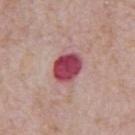<record>
  <biopsy_status>not biopsied; imaged during a skin examination</biopsy_status>
  <lesion_size>
    <long_diameter_mm_approx>3.5</long_diameter_mm_approx>
  </lesion_size>
  <image>
    <source>total-body photography crop</source>
    <field_of_view_mm>15</field_of_view_mm>
  </image>
  <lighting>white-light</lighting>
  <site>abdomen</site>
  <patient>
    <sex>male</sex>
    <age_approx>70</age_approx>
  </patient>
</record>